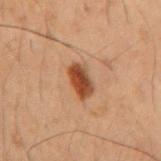Assessment:
Recorded during total-body skin imaging; not selected for excision or biopsy.
Context:
Measured at roughly 3.5 mm in maximum diameter. Automated image analysis of the tile measured a footprint of about 6 mm² and a symmetry-axis asymmetry near 0.2. A male patient aged 48 to 52. Captured under cross-polarized illumination. From the mid back. A region of skin cropped from a whole-body photographic capture, roughly 15 mm wide.